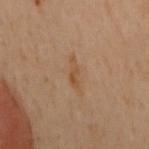{"biopsy_status": "not biopsied; imaged during a skin examination", "automated_metrics": {"cielab_L": 43, "cielab_a": 16, "cielab_b": 30, "vs_skin_darker_L": 6.0, "vs_skin_contrast_norm": 5.5, "border_irregularity_0_10": 4.5, "color_variation_0_10": 1.5, "peripheral_color_asymmetry": 0.5}, "patient": {"sex": "female", "age_approx": 60}, "lesion_size": {"long_diameter_mm_approx": 3.5}, "image": {"source": "total-body photography crop", "field_of_view_mm": 15}, "lighting": "cross-polarized", "site": "upper back"}